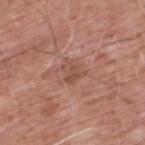Q: Is there a histopathology result?
A: imaged on a skin check; not biopsied
Q: What is the imaging modality?
A: ~15 mm tile from a whole-body skin photo
Q: Illumination type?
A: white-light illumination
Q: Who is the patient?
A: male, about 60 years old
Q: Lesion size?
A: ≈2.5 mm
Q: Where on the body is the lesion?
A: the upper back